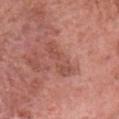Q: Is there a histopathology result?
A: imaged on a skin check; not biopsied
Q: What are the patient's age and sex?
A: female, roughly 60 years of age
Q: What lighting was used for the tile?
A: white-light illumination
Q: What kind of image is this?
A: 15 mm crop, total-body photography
Q: Lesion location?
A: the head or neck
Q: What is the lesion's diameter?
A: about 5 mm